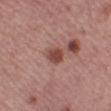automated metrics — a lesion area of about 4 mm² and an eccentricity of roughly 0.7; a border-irregularity index near 1.5/10, a color-variation rating of about 2/10, and peripheral color asymmetry of about 0.5 | body site — the left lower leg | illumination — white-light | patient — female, roughly 50 years of age | size — about 2.5 mm | acquisition — total-body-photography crop, ~15 mm field of view.